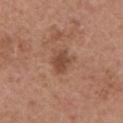{
  "biopsy_status": "not biopsied; imaged during a skin examination",
  "lesion_size": {
    "long_diameter_mm_approx": 3.0
  },
  "image": {
    "source": "total-body photography crop",
    "field_of_view_mm": 15
  },
  "site": "chest",
  "patient": {
    "sex": "male",
    "age_approx": 75
  },
  "automated_metrics": {
    "nevus_likeness_0_100": 20,
    "lesion_detection_confidence_0_100": 100
  }
}